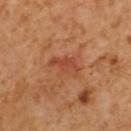The lesion was tiled from a total-body skin photograph and was not biopsied.
Imaged with cross-polarized lighting.
On the upper back.
The lesion-visualizer software estimated a footprint of about 5.5 mm², an eccentricity of roughly 0.85, and a shape-asymmetry score of about 0.4 (0 = symmetric). The analysis additionally found a border-irregularity rating of about 4.5/10 and a within-lesion color-variation index near 2/10. The analysis additionally found an automated nevus-likeness rating near 0 out of 100 and a detector confidence of about 100 out of 100 that the crop contains a lesion.
A 15 mm close-up extracted from a 3D total-body photography capture.
Longest diameter approximately 3.5 mm.
A male patient, approximately 60 years of age.